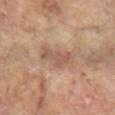Notes:
• follow-up · imaged on a skin check; not biopsied
• image source · total-body-photography crop, ~15 mm field of view
• anatomic site · the arm
• subject · female, aged 78 to 82
• diameter · about 4 mm
• illumination · cross-polarized